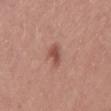Clinical impression:
This lesion was catalogued during total-body skin photography and was not selected for biopsy.
Image and clinical context:
The lesion-visualizer software estimated a border-irregularity rating of about 3.5/10, a color-variation rating of about 2/10, and radial color variation of about 0.5. From the left thigh. A female patient roughly 25 years of age. About 2.5 mm across. This image is a 15 mm lesion crop taken from a total-body photograph. This is a white-light tile.